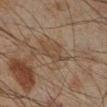follow-up: catalogued during a skin exam; not biopsied
body site: the leg
patient: male, aged 43–47
image: total-body-photography crop, ~15 mm field of view